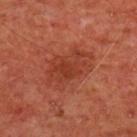notes = imaged on a skin check; not biopsied | lesion diameter = ~6.5 mm (longest diameter) | anatomic site = the front of the torso | image source = 15 mm crop, total-body photography | subject = male, aged 58 to 62.